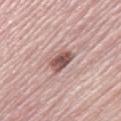Impression: Part of a total-body skin-imaging series; this lesion was reviewed on a skin check and was not flagged for biopsy. Clinical summary: A female subject aged approximately 65. The lesion is on the leg. Cropped from a total-body skin-imaging series; the visible field is about 15 mm. Longest diameter approximately 3.5 mm. Captured under white-light illumination. Automated tile analysis of the lesion measured an area of roughly 6.5 mm² and a symmetry-axis asymmetry near 0.3. It also reported a mean CIELAB color near L≈54 a*≈21 b*≈22, about 14 CIELAB-L* units darker than the surrounding skin, and a normalized border contrast of about 9.5. It also reported a border-irregularity rating of about 3/10 and a color-variation rating of about 4.5/10.